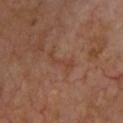| feature | finding |
|---|---|
| automated lesion analysis | a shape eccentricity near 0.95 and a symmetry-axis asymmetry near 0.55; an average lesion color of about L≈41 a*≈21 b*≈29 (CIELAB), roughly 5 lightness units darker than nearby skin, and a normalized border contrast of about 5; border irregularity of about 7.5 on a 0–10 scale, a within-lesion color-variation index near 0/10, and radial color variation of about 0; a classifier nevus-likeness of about 0/100 and a detector confidence of about 100 out of 100 that the crop contains a lesion |
| size | about 4 mm |
| subject | aged around 65 |
| illumination | cross-polarized |
| imaging modality | 15 mm crop, total-body photography |
| body site | the chest |